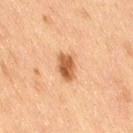Image and clinical context:
The subject is a female aged approximately 55. The lesion's longest dimension is about 3 mm. The lesion is on the right thigh. The tile uses cross-polarized illumination. Cropped from a whole-body photographic skin survey; the tile spans about 15 mm.